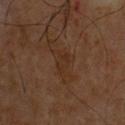Clinical impression:
The lesion was tiled from a total-body skin photograph and was not biopsied.
Background:
The recorded lesion diameter is about 3.5 mm. Cropped from a whole-body photographic skin survey; the tile spans about 15 mm. The lesion-visualizer software estimated a lesion area of about 6 mm², an eccentricity of roughly 0.85, and two-axis asymmetry of about 0.45. It also reported an automated nevus-likeness rating near 0 out of 100. Imaged with cross-polarized lighting. On the front of the torso. The subject is a male aged 58–62.